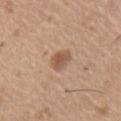workup — total-body-photography surveillance lesion; no biopsy | lesion size — ~2.5 mm (longest diameter) | subject — female, aged 73–77 | site — the chest | image source — total-body-photography crop, ~15 mm field of view.